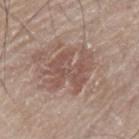Recorded during total-body skin imaging; not selected for excision or biopsy.
On the left upper arm.
Cropped from a whole-body photographic skin survey; the tile spans about 15 mm.
Approximately 5.5 mm at its widest.
A male patient approximately 75 years of age.
Captured under white-light illumination.